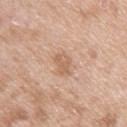Assessment: Captured during whole-body skin photography for melanoma surveillance; the lesion was not biopsied. Image and clinical context: From the left upper arm. This is a white-light tile. Cropped from a total-body skin-imaging series; the visible field is about 15 mm. The subject is a male in their 50s.